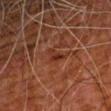notes = no biopsy performed (imaged during a skin exam)
patient = male, in their 80s
lesion size = ~2.5 mm (longest diameter)
image source = total-body-photography crop, ~15 mm field of view
site = the arm